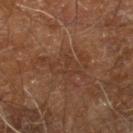No biopsy was performed on this lesion — it was imaged during a full skin examination and was not determined to be concerning. A 15 mm close-up tile from a total-body photography series done for melanoma screening. Located on the right leg. Imaged with cross-polarized lighting. The subject is a male approximately 60 years of age. Measured at roughly 6 mm in maximum diameter.